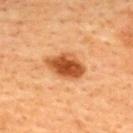The lesion was photographed on a routine skin check and not biopsied; there is no pathology result. The lesion is on the back. The lesion's longest dimension is about 5 mm. A male patient about 45 years old. A close-up tile cropped from a whole-body skin photograph, about 15 mm across. This is a cross-polarized tile.